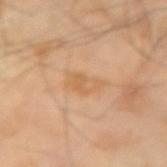* workup: catalogued during a skin exam; not biopsied
* automated lesion analysis: a border-irregularity rating of about 3.5/10, a color-variation rating of about 3.5/10, and peripheral color asymmetry of about 1; lesion-presence confidence of about 100/100
* patient: male, aged around 65
* lighting: cross-polarized
* site: the arm
* lesion size: ~4 mm (longest diameter)
* acquisition: total-body-photography crop, ~15 mm field of view